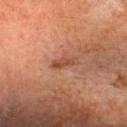The lesion was photographed on a routine skin check and not biopsied; there is no pathology result.
The lesion is located on the head or neck.
A 15 mm crop from a total-body photograph taken for skin-cancer surveillance.
The tile uses cross-polarized illumination.
The lesion's longest dimension is about 3 mm.
A female patient, aged approximately 55.